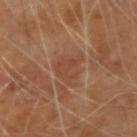biopsy status: catalogued during a skin exam; not biopsied | anatomic site: the right forearm | subject: male, aged approximately 70 | image-analysis metrics: a footprint of about 7.5 mm², an outline eccentricity of about 0.6 (0 = round, 1 = elongated), and two-axis asymmetry of about 0.45; a lesion color around L≈43 a*≈21 b*≈30 in CIELAB, roughly 6 lightness units darker than nearby skin, and a normalized lesion–skin contrast near 5; border irregularity of about 4.5 on a 0–10 scale, a color-variation rating of about 2/10, and radial color variation of about 1 | acquisition: ~15 mm crop, total-body skin-cancer survey | diameter: ~3.5 mm (longest diameter).